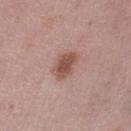Findings:
• biopsy status: total-body-photography surveillance lesion; no biopsy
• location: the left lower leg
• imaging modality: ~15 mm crop, total-body skin-cancer survey
• patient: female, aged 48–52